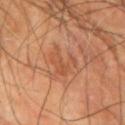Clinical impression: Captured during whole-body skin photography for melanoma surveillance; the lesion was not biopsied. Acquisition and patient details: Automated tile analysis of the lesion measured a classifier nevus-likeness of about 0/100 and a detector confidence of about 100 out of 100 that the crop contains a lesion. Longest diameter approximately 3.5 mm. A male patient aged around 65. From the left upper arm. A 15 mm close-up tile from a total-body photography series done for melanoma screening.